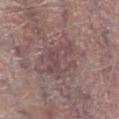The lesion was tiled from a total-body skin photograph and was not biopsied. A male patient about 55 years old. The lesion's longest dimension is about 4.5 mm. A region of skin cropped from a whole-body photographic capture, roughly 15 mm wide. Located on the right lower leg.